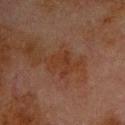The lesion was photographed on a routine skin check and not biopsied; there is no pathology result. The subject is a male aged 78–82. Cropped from a whole-body photographic skin survey; the tile spans about 15 mm. Located on the chest.